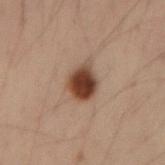Clinical impression:
No biopsy was performed on this lesion — it was imaged during a full skin examination and was not determined to be concerning.
Background:
Imaged with cross-polarized lighting. A 15 mm crop from a total-body photograph taken for skin-cancer surveillance. The lesion is located on the right forearm. A male patient about 35 years old.